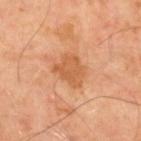A lesion tile, about 15 mm wide, cut from a 3D total-body photograph. About 4 mm across. The lesion is located on the upper back. The tile uses cross-polarized illumination. An algorithmic analysis of the crop reported an eccentricity of roughly 0.55. The analysis additionally found a border-irregularity rating of about 3/10, internal color variation of about 2 on a 0–10 scale, and a peripheral color-asymmetry measure near 0.5. It also reported lesion-presence confidence of about 100/100. The patient is a male aged 48 to 52.